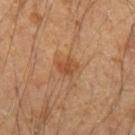{"biopsy_status": "not biopsied; imaged during a skin examination", "lesion_size": {"long_diameter_mm_approx": 2.5}, "site": "left forearm", "image": {"source": "total-body photography crop", "field_of_view_mm": 15}, "patient": {"sex": "male", "age_approx": 50}}